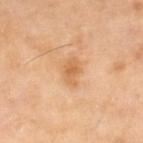Clinical impression: Part of a total-body skin-imaging series; this lesion was reviewed on a skin check and was not flagged for biopsy. Clinical summary: The subject is a female roughly 70 years of age. This image is a 15 mm lesion crop taken from a total-body photograph. Located on the right lower leg. Approximately 3.5 mm at its widest. Automated image analysis of the tile measured an area of roughly 5.5 mm² and an eccentricity of roughly 0.75. And it measured a border-irregularity index near 2.5/10, a color-variation rating of about 3/10, and radial color variation of about 1. It also reported a nevus-likeness score of about 25/100 and lesion-presence confidence of about 100/100. The tile uses cross-polarized illumination.